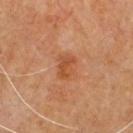Q: What did automated image analysis measure?
A: a lesion area of about 5.5 mm², an outline eccentricity of about 0.75 (0 = round, 1 = elongated), and two-axis asymmetry of about 0.2; a border-irregularity index near 2/10, a color-variation rating of about 2.5/10, and peripheral color asymmetry of about 1
Q: How large is the lesion?
A: ≈3 mm
Q: What is the imaging modality?
A: ~15 mm tile from a whole-body skin photo
Q: Illumination type?
A: cross-polarized
Q: Where on the body is the lesion?
A: the front of the torso
Q: Who is the patient?
A: male, in their 60s